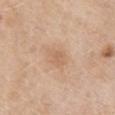biopsy status = no biopsy performed (imaged during a skin exam)
location = the mid back
image source = 15 mm crop, total-body photography
lighting = white-light illumination
patient = female, approximately 60 years of age
size = ≈3 mm